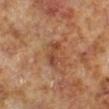Impression:
Recorded during total-body skin imaging; not selected for excision or biopsy.
Context:
The tile uses cross-polarized illumination. The subject is a male aged 58 to 62. A region of skin cropped from a whole-body photographic capture, roughly 15 mm wide. The lesion is on the left lower leg.